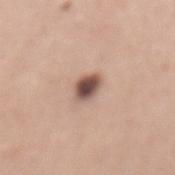Findings:
* notes · imaged on a skin check; not biopsied
* subject · female, aged 48–52
* size · about 3 mm
* image · ~15 mm tile from a whole-body skin photo
* body site · the back
* illumination · white-light